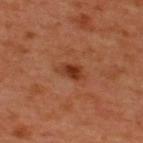Part of a total-body skin-imaging series; this lesion was reviewed on a skin check and was not flagged for biopsy.